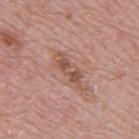workup: catalogued during a skin exam; not biopsied | location: the mid back | acquisition: ~15 mm crop, total-body skin-cancer survey | patient: male, aged 68 to 72.